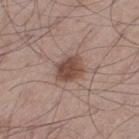The lesion was photographed on a routine skin check and not biopsied; there is no pathology result.